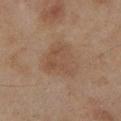Q: Is there a histopathology result?
A: no biopsy performed (imaged during a skin exam)
Q: What kind of image is this?
A: 15 mm crop, total-body photography
Q: Automated lesion metrics?
A: a lesion area of about 16 mm², a shape eccentricity near 0.25, and a shape-asymmetry score of about 0.35 (0 = symmetric); a border-irregularity index near 3/10, a within-lesion color-variation index near 2.5/10, and peripheral color asymmetry of about 1
Q: Patient demographics?
A: female, approximately 60 years of age
Q: Lesion location?
A: the left lower leg